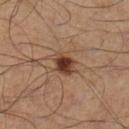follow-up — catalogued during a skin exam; not biopsied | lesion diameter — ~2.5 mm (longest diameter) | automated lesion analysis — about 15 CIELAB-L* units darker than the surrounding skin and a lesion-to-skin contrast of about 12 (normalized; higher = more distinct); a border-irregularity rating of about 2/10, internal color variation of about 4 on a 0–10 scale, and peripheral color asymmetry of about 1.5; an automated nevus-likeness rating near 95 out of 100 and a lesion-detection confidence of about 100/100 | location — the left leg | patient — male, about 50 years old | image — total-body-photography crop, ~15 mm field of view.